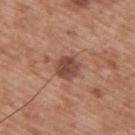Case summary:
* notes: total-body-photography surveillance lesion; no biopsy
* anatomic site: the upper back
* automated lesion analysis: a footprint of about 6.5 mm², an outline eccentricity of about 0.45 (0 = round, 1 = elongated), and a symmetry-axis asymmetry near 0.1; internal color variation of about 3.5 on a 0–10 scale and radial color variation of about 1
* lighting: white-light
* subject: male, aged approximately 50
* imaging modality: ~15 mm crop, total-body skin-cancer survey